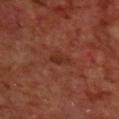Imaged during a routine full-body skin examination; the lesion was not biopsied and no histopathology is available. A male subject, aged around 70. The recorded lesion diameter is about 2.5 mm. A 15 mm crop from a total-body photograph taken for skin-cancer surveillance. This is a cross-polarized tile. Located on the upper back.